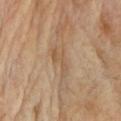* biopsy status — no biopsy performed (imaged during a skin exam)
* image source — ~15 mm tile from a whole-body skin photo
* size — ≈4 mm
* patient — female, approximately 70 years of age
* tile lighting — cross-polarized
* body site — the head or neck